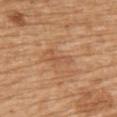Captured during whole-body skin photography for melanoma surveillance; the lesion was not biopsied.
A male patient, aged 68 to 72.
On the upper back.
The tile uses white-light illumination.
A roughly 15 mm field-of-view crop from a total-body skin photograph.